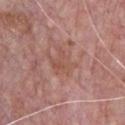Findings:
– workup · total-body-photography surveillance lesion; no biopsy
– location · the chest
– acquisition · ~15 mm crop, total-body skin-cancer survey
– tile lighting · white-light
– patient · male, about 70 years old
– lesion diameter · about 3.5 mm